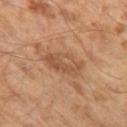The lesion was tiled from a total-body skin photograph and was not biopsied.
The total-body-photography lesion software estimated about 8 CIELAB-L* units darker than the surrounding skin and a lesion-to-skin contrast of about 6 (normalized; higher = more distinct).
A male patient, roughly 65 years of age.
A roughly 15 mm field-of-view crop from a total-body skin photograph.
Imaged with cross-polarized lighting.